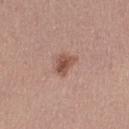A close-up tile cropped from a whole-body skin photograph, about 15 mm across. Imaged with white-light lighting. Located on the right thigh. A female subject approximately 45 years of age. About 2.5 mm across. The total-body-photography lesion software estimated border irregularity of about 3.5 on a 0–10 scale and internal color variation of about 4 on a 0–10 scale.